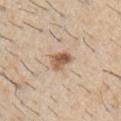This lesion was catalogued during total-body skin photography and was not selected for biopsy.
Cropped from a whole-body photographic skin survey; the tile spans about 15 mm.
Measured at roughly 3 mm in maximum diameter.
A male patient aged around 60.
The lesion-visualizer software estimated an area of roughly 5 mm², an eccentricity of roughly 0.75, and a shape-asymmetry score of about 0.25 (0 = symmetric). It also reported a lesion–skin lightness drop of about 14 and a lesion-to-skin contrast of about 9 (normalized; higher = more distinct).
The lesion is located on the right upper arm.